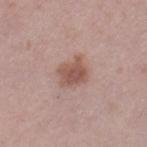Captured during whole-body skin photography for melanoma surveillance; the lesion was not biopsied.
The lesion is on the left thigh.
The subject is a female aged around 40.
This is a white-light tile.
The recorded lesion diameter is about 4 mm.
A 15 mm close-up extracted from a 3D total-body photography capture.